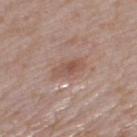notes = catalogued during a skin exam; not biopsied
location = the left thigh
subject = female, roughly 60 years of age
lesion diameter = about 3.5 mm
lighting = white-light illumination
image = ~15 mm crop, total-body skin-cancer survey